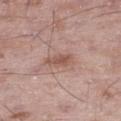Automated image analysis of the tile measured an average lesion color of about L≈54 a*≈21 b*≈25 (CIELAB), roughly 9 lightness units darker than nearby skin, and a lesion-to-skin contrast of about 7 (normalized; higher = more distinct). The tile uses white-light illumination. A male subject about 50 years old. A lesion tile, about 15 mm wide, cut from a 3D total-body photograph. Located on the leg.